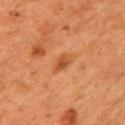Findings:
- follow-up · no biopsy performed (imaged during a skin exam)
- acquisition · 15 mm crop, total-body photography
- lighting · cross-polarized illumination
- diameter · about 2.5 mm
- anatomic site · the left upper arm
- patient · female, aged 48 to 52